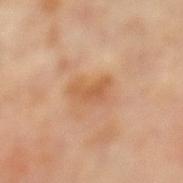Q: Is there a histopathology result?
A: no biopsy performed (imaged during a skin exam)
Q: How large is the lesion?
A: ~4 mm (longest diameter)
Q: What lighting was used for the tile?
A: cross-polarized illumination
Q: Automated lesion metrics?
A: a footprint of about 6 mm² and a shape-asymmetry score of about 0.45 (0 = symmetric); an average lesion color of about L≈58 a*≈23 b*≈38 (CIELAB) and about 8 CIELAB-L* units darker than the surrounding skin; a within-lesion color-variation index near 2.5/10 and radial color variation of about 1; an automated nevus-likeness rating near 0 out of 100
Q: What is the imaging modality?
A: ~15 mm tile from a whole-body skin photo
Q: What are the patient's age and sex?
A: female, about 60 years old
Q: What is the anatomic site?
A: the left lower leg